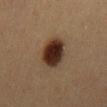biopsy status = catalogued during a skin exam; not biopsied | anatomic site = the mid back | subject = female, aged 38 to 42 | imaging modality = ~15 mm crop, total-body skin-cancer survey.